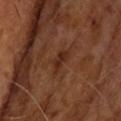A 15 mm crop from a total-body photograph taken for skin-cancer surveillance. Approximately 3 mm at its widest. Automated tile analysis of the lesion measured a footprint of about 4.5 mm², a shape eccentricity near 0.85, and two-axis asymmetry of about 0.35. And it measured a border-irregularity rating of about 4/10, a within-lesion color-variation index near 2/10, and a peripheral color-asymmetry measure near 0.5. The software also gave a classifier nevus-likeness of about 0/100. Located on the upper back. A male patient aged 68–72.